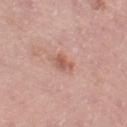This lesion was catalogued during total-body skin photography and was not selected for biopsy.